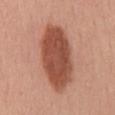<tbp_lesion>
  <biopsy_status>not biopsied; imaged during a skin examination</biopsy_status>
  <automated_metrics>
    <cielab_L>50</cielab_L>
    <cielab_a>26</cielab_a>
    <cielab_b>30</cielab_b>
    <vs_skin_darker_L>15.0</vs_skin_darker_L>
    <vs_skin_contrast_norm>10.0</vs_skin_contrast_norm>
    <color_variation_0_10>3.5</color_variation_0_10>
    <peripheral_color_asymmetry>1.0</peripheral_color_asymmetry>
  </automated_metrics>
  <image>
    <source>total-body photography crop</source>
    <field_of_view_mm>15</field_of_view_mm>
  </image>
  <patient>
    <sex>female</sex>
    <age_approx>55</age_approx>
  </patient>
  <site>mid back</site>
</tbp_lesion>